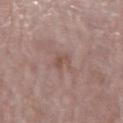The lesion was tiled from a total-body skin photograph and was not biopsied. This is a white-light tile. A female patient, aged around 70. A lesion tile, about 15 mm wide, cut from a 3D total-body photograph. Longest diameter approximately 2.5 mm. Automated image analysis of the tile measured roughly 6 lightness units darker than nearby skin and a lesion-to-skin contrast of about 5.5 (normalized; higher = more distinct). The analysis additionally found a border-irregularity index near 3.5/10, a within-lesion color-variation index near 1/10, and radial color variation of about 0.5. The lesion is located on the right upper arm.